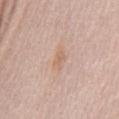Recorded during total-body skin imaging; not selected for excision or biopsy.
On the abdomen.
A close-up tile cropped from a whole-body skin photograph, about 15 mm across.
A male subject, in their mid-60s.